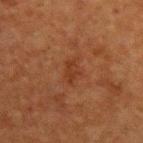Assessment: The lesion was tiled from a total-body skin photograph and was not biopsied. Context: The total-body-photography lesion software estimated a symmetry-axis asymmetry near 0.35. The software also gave about 5 CIELAB-L* units darker than the surrounding skin. The analysis additionally found border irregularity of about 3.5 on a 0–10 scale, internal color variation of about 2 on a 0–10 scale, and peripheral color asymmetry of about 1. A male patient aged 48 to 52. About 3 mm across. Located on the upper back. Imaged with cross-polarized lighting. A 15 mm close-up extracted from a 3D total-body photography capture.